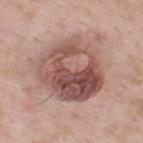Q: Was this lesion biopsied?
A: imaged on a skin check; not biopsied
Q: What kind of image is this?
A: 15 mm crop, total-body photography
Q: Where on the body is the lesion?
A: the upper back
Q: Who is the patient?
A: male, about 55 years old